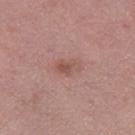<lesion>
<biopsy_status>not biopsied; imaged during a skin examination</biopsy_status>
<patient>
  <sex>female</sex>
  <age_approx>50</age_approx>
</patient>
<lighting>white-light</lighting>
<image>
  <source>total-body photography crop</source>
  <field_of_view_mm>15</field_of_view_mm>
</image>
<automated_metrics>
  <area_mm2_approx>4.5</area_mm2_approx>
  <eccentricity>0.8</eccentricity>
  <shape_asymmetry>0.25</shape_asymmetry>
  <cielab_L>52</cielab_L>
  <cielab_a>22</cielab_a>
  <cielab_b>24</cielab_b>
  <vs_skin_darker_L>8.0</vs_skin_darker_L>
  <vs_skin_contrast_norm>6.0</vs_skin_contrast_norm>
  <border_irregularity_0_10>2.5</border_irregularity_0_10>
  <peripheral_color_asymmetry>1.0</peripheral_color_asymmetry>
</automated_metrics>
<lesion_size>
  <long_diameter_mm_approx>3.0</long_diameter_mm_approx>
</lesion_size>
<site>left thigh</site>
</lesion>